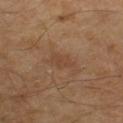The lesion was tiled from a total-body skin photograph and was not biopsied. The lesion-visualizer software estimated a border-irregularity rating of about 3/10, internal color variation of about 2 on a 0–10 scale, and a peripheral color-asymmetry measure near 0.5. About 3 mm across. This image is a 15 mm lesion crop taken from a total-body photograph. This is a cross-polarized tile. From the right lower leg. The patient is a male roughly 55 years of age.